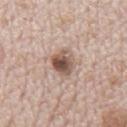| field | value |
|---|---|
| biopsy status | total-body-photography surveillance lesion; no biopsy |
| acquisition | ~15 mm tile from a whole-body skin photo |
| anatomic site | the mid back |
| subject | male, aged approximately 70 |
| illumination | white-light |
| automated metrics | a footprint of about 8 mm² and an eccentricity of roughly 0.65; a lesion color around L≈54 a*≈17 b*≈25 in CIELAB, a lesion–skin lightness drop of about 15, and a normalized lesion–skin contrast near 10; a color-variation rating of about 8.5/10 and peripheral color asymmetry of about 3 |